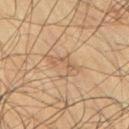{"biopsy_status": "not biopsied; imaged during a skin examination", "lesion_size": {"long_diameter_mm_approx": 4.0}, "image": {"source": "total-body photography crop", "field_of_view_mm": 15}, "patient": {"sex": "male", "age_approx": 75}, "automated_metrics": {"area_mm2_approx": 5.0, "eccentricity": 0.9, "shape_asymmetry": 0.5, "cielab_L": 49, "cielab_a": 15, "cielab_b": 29, "vs_skin_darker_L": 6.0, "vs_skin_contrast_norm": 5.0, "color_variation_0_10": 2.0, "peripheral_color_asymmetry": 0.5, "nevus_likeness_0_100": 0, "lesion_detection_confidence_0_100": 90}, "site": "right upper arm", "lighting": "cross-polarized"}